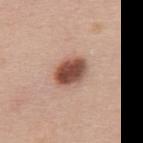* notes · imaged on a skin check; not biopsied
* tile lighting · white-light illumination
* image · ~15 mm tile from a whole-body skin photo
* lesion size · ≈4 mm
* location · the upper back
* subject · female, about 40 years old